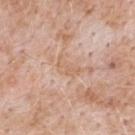Clinical impression: Captured during whole-body skin photography for melanoma surveillance; the lesion was not biopsied. Context: From the upper back. A male patient, roughly 50 years of age. An algorithmic analysis of the crop reported an area of roughly 4 mm² and a shape eccentricity near 0.85. The software also gave border irregularity of about 4.5 on a 0–10 scale. A region of skin cropped from a whole-body photographic capture, roughly 15 mm wide. The lesion's longest dimension is about 3 mm.